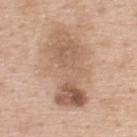follow-up: total-body-photography surveillance lesion; no biopsy | site: the upper back | subject: male, aged 48–52 | image source: ~15 mm tile from a whole-body skin photo.